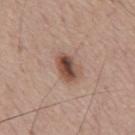This lesion was catalogued during total-body skin photography and was not selected for biopsy. The lesion's longest dimension is about 3.5 mm. A lesion tile, about 15 mm wide, cut from a 3D total-body photograph. This is a white-light tile. A male patient approximately 55 years of age.